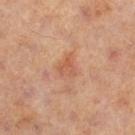Image and clinical context: Cropped from a total-body skin-imaging series; the visible field is about 15 mm. Captured under cross-polarized illumination. A male subject aged around 60. From the left thigh. The recorded lesion diameter is about 2.5 mm.